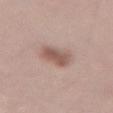biopsy_status: not biopsied; imaged during a skin examination